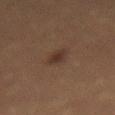Notes:
– site — the back
– automated lesion analysis — a border-irregularity rating of about 2/10; a nevus-likeness score of about 75/100
– image source — ~15 mm tile from a whole-body skin photo
– subject — female, aged 68–72
– tile lighting — cross-polarized